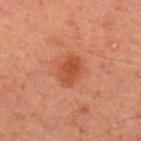follow-up: no biopsy performed (imaged during a skin exam)
patient: male, roughly 45 years of age
acquisition: total-body-photography crop, ~15 mm field of view
anatomic site: the upper back